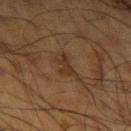Case summary:
– notes — catalogued during a skin exam; not biopsied
– image source — 15 mm crop, total-body photography
– location — the left upper arm
– subject — male, aged 58 to 62